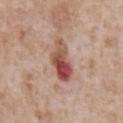Image and clinical context: A close-up tile cropped from a whole-body skin photograph, about 15 mm across. A male patient, aged 63 to 67. Located on the front of the torso.